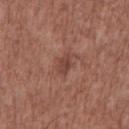Part of a total-body skin-imaging series; this lesion was reviewed on a skin check and was not flagged for biopsy. On the chest. The tile uses white-light illumination. A male subject, roughly 75 years of age. A region of skin cropped from a whole-body photographic capture, roughly 15 mm wide.